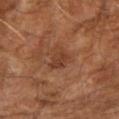{
  "patient": {
    "sex": "male",
    "age_approx": 65
  },
  "lighting": "cross-polarized",
  "site": "right upper arm",
  "lesion_size": {
    "long_diameter_mm_approx": 3.0
  },
  "image": {
    "source": "total-body photography crop",
    "field_of_view_mm": 15
  }
}